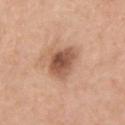<record>
<biopsy_status>not biopsied; imaged during a skin examination</biopsy_status>
<site>chest</site>
<image>
  <source>total-body photography crop</source>
  <field_of_view_mm>15</field_of_view_mm>
</image>
<automated_metrics>
  <eccentricity>0.35</eccentricity>
  <shape_asymmetry>0.25</shape_asymmetry>
  <cielab_L>55</cielab_L>
  <cielab_a>22</cielab_a>
  <cielab_b>31</cielab_b>
</automated_metrics>
<patient>
  <sex>male</sex>
  <age_approx>40</age_approx>
</patient>
<lesion_size>
  <long_diameter_mm_approx>4.0</long_diameter_mm_approx>
</lesion_size>
<lighting>white-light</lighting>
</record>